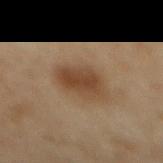Assessment:
The lesion was tiled from a total-body skin photograph and was not biopsied.
Clinical summary:
A female subject, aged around 60. A 15 mm close-up extracted from a 3D total-body photography capture. The lesion is on the back.